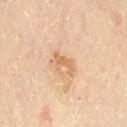{
  "biopsy_status": "not biopsied; imaged during a skin examination",
  "patient": {
    "sex": "female",
    "age_approx": 60
  },
  "automated_metrics": {
    "shape_asymmetry": 0.65,
    "cielab_L": 66,
    "cielab_a": 20,
    "cielab_b": 39,
    "vs_skin_darker_L": 9.0,
    "vs_skin_contrast_norm": 6.5,
    "border_irregularity_0_10": 7.0,
    "color_variation_0_10": 0.0,
    "peripheral_color_asymmetry": 0.0
  },
  "site": "lower back",
  "image": {
    "source": "total-body photography crop",
    "field_of_view_mm": 15
  },
  "lighting": "cross-polarized"
}